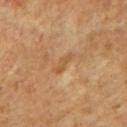follow-up: no biopsy performed (imaged during a skin exam); illumination: cross-polarized; body site: the left upper arm; image source: ~15 mm crop, total-body skin-cancer survey; patient: female, aged approximately 60.